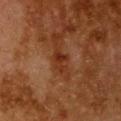<lesion>
  <image>
    <source>total-body photography crop</source>
    <field_of_view_mm>15</field_of_view_mm>
  </image>
  <site>chest</site>
  <patient>
    <sex>female</sex>
    <age_approx>50</age_approx>
  </patient>
</lesion>